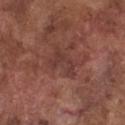follow-up: catalogued during a skin exam; not biopsied | anatomic site: the chest | lesion diameter: ~4 mm (longest diameter) | subject: male, aged 73–77 | illumination: white-light | imaging modality: 15 mm crop, total-body photography.